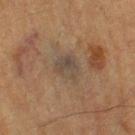notes=no biopsy performed (imaged during a skin exam)
lighting=cross-polarized
automated lesion analysis=a border-irregularity rating of about 2.5/10, a within-lesion color-variation index near 5/10, and a peripheral color-asymmetry measure near 2
patient=male, roughly 85 years of age or older
imaging modality=15 mm crop, total-body photography
site=the left thigh